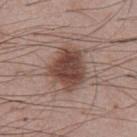This lesion was catalogued during total-body skin photography and was not selected for biopsy. A male subject approximately 55 years of age. A region of skin cropped from a whole-body photographic capture, roughly 15 mm wide. Captured under white-light illumination. On the chest. About 5 mm across.